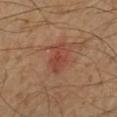Recorded during total-body skin imaging; not selected for excision or biopsy.
On the left lower leg.
Longest diameter approximately 4 mm.
The total-body-photography lesion software estimated border irregularity of about 3.5 on a 0–10 scale, a color-variation rating of about 2.5/10, and radial color variation of about 1. The software also gave a classifier nevus-likeness of about 55/100 and a lesion-detection confidence of about 100/100.
A male patient aged approximately 55.
Cropped from a total-body skin-imaging series; the visible field is about 15 mm.